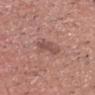Notes:
• image — 15 mm crop, total-body photography
• illumination — white-light
• site — the head or neck
• image-analysis metrics — a lesion area of about 4.5 mm², an outline eccentricity of about 0.7 (0 = round, 1 = elongated), and a symmetry-axis asymmetry near 0.4; an average lesion color of about L≈50 a*≈21 b*≈24 (CIELAB) and a normalized lesion–skin contrast near 6; a border-irregularity index near 4/10
• patient — male, in their mid-50s
• diameter — ~3 mm (longest diameter)